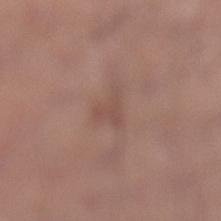Imaged during a routine full-body skin examination; the lesion was not biopsied and no histopathology is available. This is a white-light tile. The lesion is on the leg. Automated tile analysis of the lesion measured an area of roughly 4.5 mm², a shape eccentricity near 0.55, and a shape-asymmetry score of about 0.55 (0 = symmetric). The software also gave a border-irregularity index near 5.5/10, internal color variation of about 1 on a 0–10 scale, and peripheral color asymmetry of about 0.5. The analysis additionally found a nevus-likeness score of about 0/100 and a lesion-detection confidence of about 95/100. A male subject, approximately 55 years of age. Longest diameter approximately 3 mm. A 15 mm close-up tile from a total-body photography series done for melanoma screening.